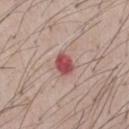Clinical impression:
Recorded during total-body skin imaging; not selected for excision or biopsy.
Acquisition and patient details:
Captured under white-light illumination. A region of skin cropped from a whole-body photographic capture, roughly 15 mm wide. The patient is a male aged 53–57.